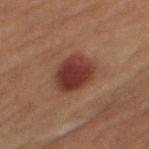The lesion was tiled from a total-body skin photograph and was not biopsied. About 4.5 mm across. A female patient, aged around 80. A 15 mm close-up tile from a total-body photography series done for melanoma screening. On the right thigh.